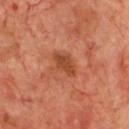subject: male, in their 70s
anatomic site: the chest
image: total-body-photography crop, ~15 mm field of view
diameter: ≈3.5 mm